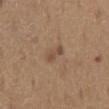Assessment:
No biopsy was performed on this lesion — it was imaged during a full skin examination and was not determined to be concerning.